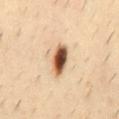  image:
    source: total-body photography crop
    field_of_view_mm: 15
  patient:
    sex: male
    age_approx: 40
  lesion_size:
    long_diameter_mm_approx: 4.0
  site: mid back
  lighting: cross-polarized